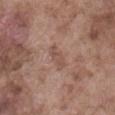No biopsy was performed on this lesion — it was imaged during a full skin examination and was not determined to be concerning.
An algorithmic analysis of the crop reported a lesion area of about 4 mm² and an eccentricity of roughly 0.9. It also reported a border-irregularity rating of about 3.5/10, a color-variation rating of about 2/10, and radial color variation of about 1.
The lesion is located on the abdomen.
The subject is a male aged 73 to 77.
This image is a 15 mm lesion crop taken from a total-body photograph.
Captured under white-light illumination.